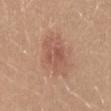The lesion was photographed on a routine skin check and not biopsied; there is no pathology result.
A roughly 15 mm field-of-view crop from a total-body skin photograph.
The lesion is on the back.
The lesion-visualizer software estimated roughly 7 lightness units darker than nearby skin. The software also gave a color-variation rating of about 4/10 and peripheral color asymmetry of about 1.5.
This is a white-light tile.
A female subject aged 28 to 32.
About 4 mm across.